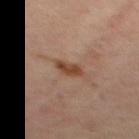anatomic site = the mid back
subject = female, about 45 years old
illumination = cross-polarized
automated lesion analysis = a border-irregularity index near 3/10, a color-variation rating of about 2/10, and radial color variation of about 1; a nevus-likeness score of about 80/100 and a lesion-detection confidence of about 100/100
image source = ~15 mm crop, total-body skin-cancer survey